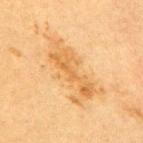Longest diameter approximately 8 mm. On the mid back. The total-body-photography lesion software estimated a footprint of about 16 mm², an eccentricity of roughly 0.95, and a shape-asymmetry score of about 0.45 (0 = symmetric). The analysis additionally found a mean CIELAB color near L≈57 a*≈19 b*≈41, a lesion–skin lightness drop of about 8, and a normalized border contrast of about 6.5. A 15 mm close-up tile from a total-body photography series done for melanoma screening. A female subject, aged 53–57.